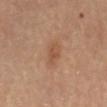Imaged during a routine full-body skin examination; the lesion was not biopsied and no histopathology is available. A roughly 15 mm field-of-view crop from a total-body skin photograph. The patient is a male aged 83 or older. An algorithmic analysis of the crop reported a lesion area of about 5 mm², an outline eccentricity of about 0.85 (0 = round, 1 = elongated), and a shape-asymmetry score of about 0.3 (0 = symmetric). The analysis additionally found a lesion–skin lightness drop of about 7 and a normalized lesion–skin contrast near 5.5. The software also gave lesion-presence confidence of about 100/100. About 3.5 mm across. The tile uses cross-polarized illumination. On the mid back.